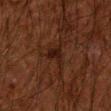workup: no biopsy performed (imaged during a skin exam)
site: the right forearm
patient: male, aged around 60
image source: total-body-photography crop, ~15 mm field of view